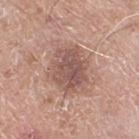The lesion was photographed on a routine skin check and not biopsied; there is no pathology result. A lesion tile, about 15 mm wide, cut from a 3D total-body photograph. A male patient approximately 80 years of age. The tile uses white-light illumination. About 5 mm across. The lesion is on the right thigh.